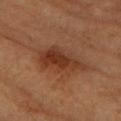Notes:
• workup — no biopsy performed (imaged during a skin exam)
• TBP lesion metrics — a footprint of about 13 mm², an outline eccentricity of about 0.9 (0 = round, 1 = elongated), and two-axis asymmetry of about 0.3; a mean CIELAB color near L≈35 a*≈23 b*≈31, about 10 CIELAB-L* units darker than the surrounding skin, and a normalized border contrast of about 8.5; an automated nevus-likeness rating near 85 out of 100 and a lesion-detection confidence of about 100/100
• patient — female, in their mid-60s
• lighting — cross-polarized illumination
• lesion size — about 6 mm
• site — the arm
• image — ~15 mm crop, total-body skin-cancer survey